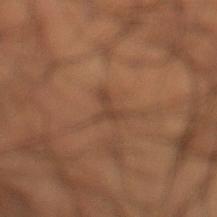notes — catalogued during a skin exam; not biopsied | illumination — cross-polarized | lesion size — ≈2.5 mm | site — the left lower leg | image source — ~15 mm tile from a whole-body skin photo | image-analysis metrics — a lesion area of about 3 mm² and a symmetry-axis asymmetry near 0.65; border irregularity of about 7 on a 0–10 scale, a within-lesion color-variation index near 0/10, and peripheral color asymmetry of about 0 | subject — male, aged 48–52.